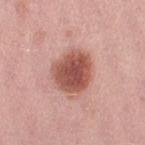Assessment:
No biopsy was performed on this lesion — it was imaged during a full skin examination and was not determined to be concerning.
Context:
Cropped from a whole-body photographic skin survey; the tile spans about 15 mm. The lesion-visualizer software estimated a lesion area of about 16 mm². It also reported an average lesion color of about L≈53 a*≈26 b*≈27 (CIELAB), roughly 15 lightness units darker than nearby skin, and a normalized border contrast of about 10. The analysis additionally found an automated nevus-likeness rating near 100 out of 100 and a detector confidence of about 100 out of 100 that the crop contains a lesion. A female patient aged 38 to 42. Captured under white-light illumination. Approximately 5 mm at its widest. On the right thigh.